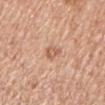notes = total-body-photography surveillance lesion; no biopsy | site = the left upper arm | diameter = ~3 mm (longest diameter) | automated lesion analysis = a lesion–skin lightness drop of about 10 and a normalized border contrast of about 6 | illumination = white-light illumination | patient = male, roughly 65 years of age | acquisition = 15 mm crop, total-body photography.